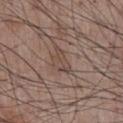<case>
  <biopsy_status>not biopsied; imaged during a skin examination</biopsy_status>
  <patient>
    <sex>male</sex>
    <age_approx>55</age_approx>
  </patient>
  <image>
    <source>total-body photography crop</source>
    <field_of_view_mm>15</field_of_view_mm>
  </image>
  <site>front of the torso</site>
</case>